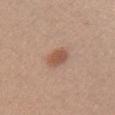Notes:
* notes — imaged on a skin check; not biopsied
* image source — ~15 mm tile from a whole-body skin photo
* TBP lesion metrics — an area of roughly 5 mm², an outline eccentricity of about 0.7 (0 = round, 1 = elongated), and a shape-asymmetry score of about 0.2 (0 = symmetric); a lesion color around L≈54 a*≈22 b*≈30 in CIELAB and a lesion-to-skin contrast of about 7.5 (normalized; higher = more distinct); an automated nevus-likeness rating near 100 out of 100
* patient — female, about 25 years old
* anatomic site — the chest
* tile lighting — white-light
* lesion diameter — ≈3 mm